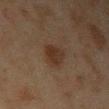A lesion tile, about 15 mm wide, cut from a 3D total-body photograph. About 4 mm across. The patient is a male roughly 45 years of age. Located on the right upper arm. This is a cross-polarized tile.